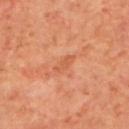Q: Was a biopsy performed?
A: no biopsy performed (imaged during a skin exam)
Q: Where on the body is the lesion?
A: the upper back
Q: What did automated image analysis measure?
A: border irregularity of about 3 on a 0–10 scale, a within-lesion color-variation index near 1/10, and peripheral color asymmetry of about 0.5; an automated nevus-likeness rating near 0 out of 100
Q: Who is the patient?
A: male, in their 70s
Q: How large is the lesion?
A: about 2.5 mm
Q: How was this image acquired?
A: total-body-photography crop, ~15 mm field of view